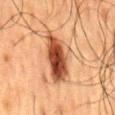notes: catalogued during a skin exam; not biopsied | body site: the mid back | acquisition: ~15 mm tile from a whole-body skin photo | subject: male, roughly 60 years of age | lesion diameter: about 7 mm | image-analysis metrics: a lesion area of about 18 mm², a shape eccentricity near 0.85, and two-axis asymmetry of about 0.25; an average lesion color of about L≈41 a*≈22 b*≈30 (CIELAB) and roughly 15 lightness units darker than nearby skin; a classifier nevus-likeness of about 100/100 | tile lighting: cross-polarized.